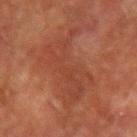The lesion was photographed on a routine skin check and not biopsied; there is no pathology result. Approximately 7 mm at its widest. A 15 mm crop from a total-body photograph taken for skin-cancer surveillance. A male subject, in their mid-70s. Located on the left upper arm. Imaged with cross-polarized lighting.